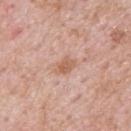A male subject aged around 55. A 15 mm close-up tile from a total-body photography series done for melanoma screening. Measured at roughly 3 mm in maximum diameter. This is a white-light tile. Located on the upper back. An algorithmic analysis of the crop reported an area of roughly 4 mm², a shape eccentricity near 0.7, and a shape-asymmetry score of about 0.3 (0 = symmetric). It also reported a mean CIELAB color near L≈60 a*≈22 b*≈31, about 9 CIELAB-L* units darker than the surrounding skin, and a normalized border contrast of about 6.5. The software also gave internal color variation of about 3 on a 0–10 scale and peripheral color asymmetry of about 1. The analysis additionally found an automated nevus-likeness rating near 10 out of 100 and lesion-presence confidence of about 100/100.